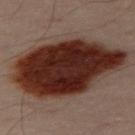Notes:
– biopsy status: total-body-photography surveillance lesion; no biopsy
– anatomic site: the left leg
– imaging modality: ~15 mm crop, total-body skin-cancer survey
– patient: male, aged approximately 50